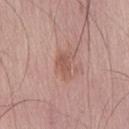Clinical impression: The lesion was photographed on a routine skin check and not biopsied; there is no pathology result. Context: The patient is a male aged approximately 60. A roughly 15 mm field-of-view crop from a total-body skin photograph. Located on the back.